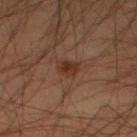workup — no biopsy performed (imaged during a skin exam); imaging modality — total-body-photography crop, ~15 mm field of view; patient — male, about 45 years old; body site — the leg.